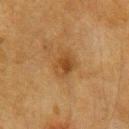Q: Is there a histopathology result?
A: imaged on a skin check; not biopsied
Q: Automated lesion metrics?
A: an area of roughly 6 mm², a shape eccentricity near 0.75, and a symmetry-axis asymmetry near 0.15; about 7 CIELAB-L* units darker than the surrounding skin and a normalized border contrast of about 6.5
Q: What lighting was used for the tile?
A: cross-polarized
Q: What is the lesion's diameter?
A: ≈3.5 mm
Q: What are the patient's age and sex?
A: female, aged 53–57
Q: What is the imaging modality?
A: ~15 mm tile from a whole-body skin photo
Q: Where on the body is the lesion?
A: the right forearm